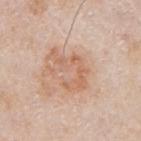Part of a total-body skin-imaging series; this lesion was reviewed on a skin check and was not flagged for biopsy. Located on the right upper arm. The lesion-visualizer software estimated about 8 CIELAB-L* units darker than the surrounding skin and a lesion-to-skin contrast of about 5.5 (normalized; higher = more distinct). And it measured a classifier nevus-likeness of about 0/100 and a lesion-detection confidence of about 100/100. Longest diameter approximately 5.5 mm. The patient is a male approximately 75 years of age. A 15 mm close-up tile from a total-body photography series done for melanoma screening.